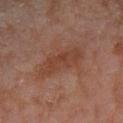This lesion was catalogued during total-body skin photography and was not selected for biopsy. Approximately 5.5 mm at its widest. The patient is a female aged approximately 60. The lesion is on the right lower leg. A region of skin cropped from a whole-body photographic capture, roughly 15 mm wide.